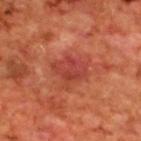Recorded during total-body skin imaging; not selected for excision or biopsy. A roughly 15 mm field-of-view crop from a total-body skin photograph. The patient is a male about 70 years old. The lesion is on the upper back. About 3.5 mm across. An algorithmic analysis of the crop reported a lesion area of about 9 mm² and a shape eccentricity near 0.6. The software also gave roughly 8 lightness units darker than nearby skin and a normalized border contrast of about 6.5. The analysis additionally found a color-variation rating of about 3.5/10 and radial color variation of about 1. The analysis additionally found a classifier nevus-likeness of about 85/100 and lesion-presence confidence of about 100/100. The tile uses cross-polarized illumination.